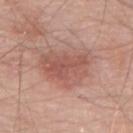biopsy status: catalogued during a skin exam; not biopsied | illumination: white-light | lesion diameter: about 6.5 mm | imaging modality: ~15 mm tile from a whole-body skin photo | patient: male, roughly 70 years of age | location: the upper back.